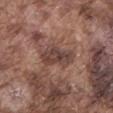Findings:
– biopsy status: imaged on a skin check; not biopsied
– imaging modality: ~15 mm tile from a whole-body skin photo
– illumination: white-light illumination
– automated lesion analysis: a footprint of about 9 mm², an outline eccentricity of about 0.8 (0 = round, 1 = elongated), and two-axis asymmetry of about 0.35; a mean CIELAB color near L≈42 a*≈19 b*≈22, a lesion–skin lightness drop of about 10, and a normalized lesion–skin contrast near 8; a nevus-likeness score of about 0/100
– size: about 4.5 mm
– patient: male, aged 73 to 77
– body site: the back